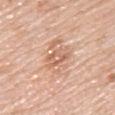Q: Is there a histopathology result?
A: total-body-photography surveillance lesion; no biopsy
Q: Who is the patient?
A: female, about 65 years old
Q: Lesion location?
A: the back
Q: What is the imaging modality?
A: ~15 mm crop, total-body skin-cancer survey